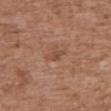Captured during whole-body skin photography for melanoma surveillance; the lesion was not biopsied. A female patient, about 75 years old. The recorded lesion diameter is about 3 mm. This is a white-light tile. A 15 mm close-up extracted from a 3D total-body photography capture. The lesion is located on the upper back. Automated tile analysis of the lesion measured a lesion area of about 3 mm², an eccentricity of roughly 0.9, and a symmetry-axis asymmetry near 0.25. It also reported an average lesion color of about L≈48 a*≈21 b*≈30 (CIELAB), roughly 7 lightness units darker than nearby skin, and a normalized border contrast of about 5.5. It also reported border irregularity of about 3 on a 0–10 scale, internal color variation of about 2 on a 0–10 scale, and radial color variation of about 1. The analysis additionally found an automated nevus-likeness rating near 0 out of 100.